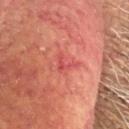Impression: Imaged during a routine full-body skin examination; the lesion was not biopsied and no histopathology is available. Background: The patient is a male aged around 55. A close-up tile cropped from a whole-body skin photograph, about 15 mm across. On the head or neck.